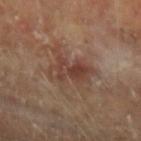Captured during whole-body skin photography for melanoma surveillance; the lesion was not biopsied.